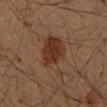Assessment: The lesion was tiled from a total-body skin photograph and was not biopsied. Background: The patient is a male aged 48–52. A close-up tile cropped from a whole-body skin photograph, about 15 mm across. Imaged with cross-polarized lighting. The total-body-photography lesion software estimated a mean CIELAB color near L≈30 a*≈18 b*≈25, a lesion–skin lightness drop of about 8, and a lesion-to-skin contrast of about 8 (normalized; higher = more distinct). And it measured a border-irregularity rating of about 3.5/10, a within-lesion color-variation index near 3.5/10, and peripheral color asymmetry of about 1. The recorded lesion diameter is about 5 mm. The lesion is on the right upper arm.